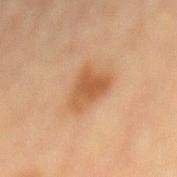  biopsy_status: not biopsied; imaged during a skin examination
  lesion_size:
    long_diameter_mm_approx: 4.5
  image:
    source: total-body photography crop
    field_of_view_mm: 15
  site: back
  patient:
    sex: female
    age_approx: 80